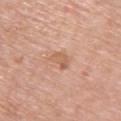follow-up: total-body-photography surveillance lesion; no biopsy
imaging modality: ~15 mm crop, total-body skin-cancer survey
subject: female, approximately 65 years of age
lesion diameter: ≈2.5 mm
lighting: white-light
anatomic site: the back